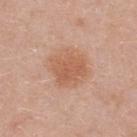  biopsy_status: not biopsied; imaged during a skin examination
  patient:
    sex: female
    age_approx: 40
  automated_metrics:
    area_mm2_approx: 17.0
    eccentricity: 0.35
    cielab_L: 60
    cielab_a: 22
    cielab_b: 32
    vs_skin_darker_L: 8.0
  lesion_size:
    long_diameter_mm_approx: 5.0
  lighting: white-light
  site: upper back
  image:
    source: total-body photography crop
    field_of_view_mm: 15